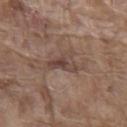{"biopsy_status": "not biopsied; imaged during a skin examination", "image": {"source": "total-body photography crop", "field_of_view_mm": 15}, "patient": {"sex": "male", "age_approx": 80}, "site": "chest"}